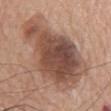notes: total-body-photography surveillance lesion; no biopsy | acquisition: ~15 mm tile from a whole-body skin photo | lighting: white-light illumination | location: the front of the torso | patient: male, roughly 75 years of age.